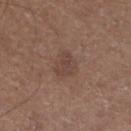Recorded during total-body skin imaging; not selected for excision or biopsy. A male patient aged 73–77. On the left lower leg. A 15 mm crop from a total-body photograph taken for skin-cancer surveillance. The lesion's longest dimension is about 3.5 mm. Automated tile analysis of the lesion measured a footprint of about 6.5 mm², a shape eccentricity near 0.7, and two-axis asymmetry of about 0.3. The software also gave a border-irregularity rating of about 3/10, internal color variation of about 2 on a 0–10 scale, and a peripheral color-asymmetry measure near 0.5. The software also gave a lesion-detection confidence of about 100/100. Imaged with white-light lighting.